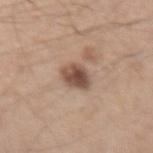Q: Was a biopsy performed?
A: no biopsy performed (imaged during a skin exam)
Q: What is the anatomic site?
A: the left upper arm
Q: What kind of image is this?
A: total-body-photography crop, ~15 mm field of view
Q: What is the lesion's diameter?
A: ≈3 mm
Q: Patient demographics?
A: male, aged 43–47
Q: What lighting was used for the tile?
A: white-light